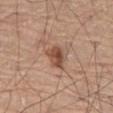workup — imaged on a skin check; not biopsied | anatomic site — the right leg | imaging modality — ~15 mm tile from a whole-body skin photo | automated lesion analysis — a mean CIELAB color near L≈50 a*≈21 b*≈29 and a lesion-to-skin contrast of about 8.5 (normalized; higher = more distinct); a detector confidence of about 100 out of 100 that the crop contains a lesion | lesion diameter — ~3.5 mm (longest diameter) | subject — male, in their 80s.